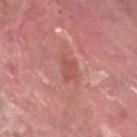Q: Is there a histopathology result?
A: total-body-photography surveillance lesion; no biopsy
Q: Automated lesion metrics?
A: an area of roughly 4.5 mm², an eccentricity of roughly 0.7, and a shape-asymmetry score of about 0.25 (0 = symmetric); a nevus-likeness score of about 0/100 and a lesion-detection confidence of about 100/100
Q: Illumination type?
A: white-light illumination
Q: How was this image acquired?
A: ~15 mm crop, total-body skin-cancer survey
Q: Lesion size?
A: about 3 mm
Q: Who is the patient?
A: male, aged around 40
Q: What is the anatomic site?
A: the left lower leg